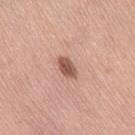follow-up — catalogued during a skin exam; not biopsied
image — total-body-photography crop, ~15 mm field of view
automated lesion analysis — an outline eccentricity of about 0.8 (0 = round, 1 = elongated); a lesion color around L≈54 a*≈23 b*≈27 in CIELAB and a lesion–skin lightness drop of about 15; a nevus-likeness score of about 90/100 and lesion-presence confidence of about 100/100
lighting — white-light illumination
subject — female, about 65 years old
anatomic site — the right thigh